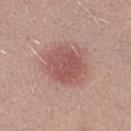Recorded during total-body skin imaging; not selected for excision or biopsy. Imaged with white-light lighting. A roughly 15 mm field-of-view crop from a total-body skin photograph. A female subject in their mid- to late 20s. The total-body-photography lesion software estimated an area of roughly 19 mm² and a symmetry-axis asymmetry near 0.15. And it measured a mean CIELAB color near L≈54 a*≈24 b*≈23, a lesion–skin lightness drop of about 10, and a lesion-to-skin contrast of about 6.5 (normalized; higher = more distinct). The software also gave a border-irregularity index near 2.5/10, a color-variation rating of about 3.5/10, and radial color variation of about 1. The analysis additionally found an automated nevus-likeness rating near 100 out of 100 and a lesion-detection confidence of about 100/100. About 5.5 mm across. From the right thigh.